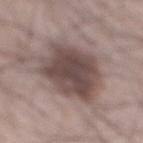Q: Was a biopsy performed?
A: no biopsy performed (imaged during a skin exam)
Q: Lesion size?
A: ≈9.5 mm
Q: Automated lesion metrics?
A: a lesion area of about 32 mm² and two-axis asymmetry of about 0.15; an average lesion color of about L≈47 a*≈14 b*≈18 (CIELAB), roughly 14 lightness units darker than nearby skin, and a normalized lesion–skin contrast near 10; a border-irregularity rating of about 2.5/10, a within-lesion color-variation index near 5/10, and peripheral color asymmetry of about 1.5
Q: Lesion location?
A: the mid back
Q: What kind of image is this?
A: 15 mm crop, total-body photography
Q: Patient demographics?
A: male, aged 63–67
Q: What lighting was used for the tile?
A: white-light